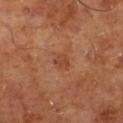{
  "site": "right lower leg",
  "automated_metrics": {
    "area_mm2_approx": 4.0,
    "eccentricity": 0.65,
    "shape_asymmetry": 0.2,
    "cielab_L": 45,
    "cielab_a": 25,
    "cielab_b": 33,
    "vs_skin_darker_L": 7.0,
    "vs_skin_contrast_norm": 5.5,
    "border_irregularity_0_10": 1.5,
    "color_variation_0_10": 3.0,
    "peripheral_color_asymmetry": 1.0,
    "nevus_likeness_0_100": 15,
    "lesion_detection_confidence_0_100": 100
  },
  "lesion_size": {
    "long_diameter_mm_approx": 2.5
  },
  "patient": {
    "sex": "male",
    "age_approx": 65
  },
  "image": {
    "source": "total-body photography crop",
    "field_of_view_mm": 15
  }
}